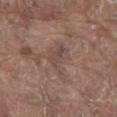workup = no biopsy performed (imaged during a skin exam)
image source = ~15 mm crop, total-body skin-cancer survey
patient = male, aged approximately 80
site = the abdomen
size = ≈5 mm
automated lesion analysis = a footprint of about 9.5 mm²; an average lesion color of about L≈47 a*≈16 b*≈22 (CIELAB), a lesion–skin lightness drop of about 6, and a normalized lesion–skin contrast near 5; a classifier nevus-likeness of about 0/100